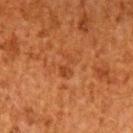The lesion is on the left upper arm.
A male subject, roughly 60 years of age.
A 15 mm crop from a total-body photograph taken for skin-cancer surveillance.